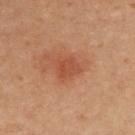This is a cross-polarized tile. Cropped from a total-body skin-imaging series; the visible field is about 15 mm. A female subject in their mid-40s. The lesion is on the back.